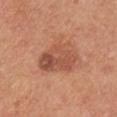Acquisition and patient details:
The patient is a male aged 28–32. Captured under white-light illumination. The lesion's longest dimension is about 5.5 mm. A close-up tile cropped from a whole-body skin photograph, about 15 mm across. The lesion is on the right upper arm.